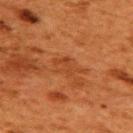Part of a total-body skin-imaging series; this lesion was reviewed on a skin check and was not flagged for biopsy.
The lesion-visualizer software estimated an automated nevus-likeness rating near 0 out of 100 and lesion-presence confidence of about 100/100.
A female patient in their 50s.
The lesion is on the upper back.
Measured at roughly 4 mm in maximum diameter.
A 15 mm crop from a total-body photograph taken for skin-cancer surveillance.
The tile uses cross-polarized illumination.